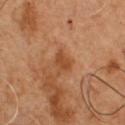workup — imaged on a skin check; not biopsied | image source — 15 mm crop, total-body photography | automated metrics — a lesion area of about 4 mm², an outline eccentricity of about 0.75 (0 = round, 1 = elongated), and a symmetry-axis asymmetry near 0.35; a nevus-likeness score of about 0/100 and a lesion-detection confidence of about 100/100 | patient — male, aged around 50 | site — the chest | lesion size — ~2.5 mm (longest diameter).